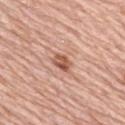– subject · female, approximately 65 years of age
– location · the upper back
– acquisition · ~15 mm tile from a whole-body skin photo
– lesion size · about 2.5 mm
– tile lighting · white-light illumination